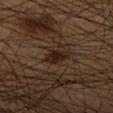workup: total-body-photography surveillance lesion; no biopsy
lesion diameter: ≈3 mm
image source: ~15 mm crop, total-body skin-cancer survey
tile lighting: cross-polarized illumination
anatomic site: the right lower leg
patient: male, in their mid- to late 40s